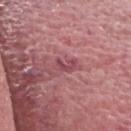Impression: No biopsy was performed on this lesion — it was imaged during a full skin examination and was not determined to be concerning. Context: The patient is a male in their mid- to late 60s. The tile uses white-light illumination. A roughly 15 mm field-of-view crop from a total-body skin photograph. The lesion is on the head or neck. The total-body-photography lesion software estimated a lesion color around L≈47 a*≈30 b*≈16 in CIELAB, roughly 9 lightness units darker than nearby skin, and a lesion-to-skin contrast of about 6.5 (normalized; higher = more distinct). The analysis additionally found a classifier nevus-likeness of about 0/100.